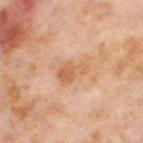Imaged during a routine full-body skin examination; the lesion was not biopsied and no histopathology is available.
Captured under cross-polarized illumination.
A 15 mm close-up tile from a total-body photography series done for melanoma screening.
The lesion is located on the right thigh.
Measured at roughly 3.5 mm in maximum diameter.
An algorithmic analysis of the crop reported an outline eccentricity of about 0.75 (0 = round, 1 = elongated). The analysis additionally found roughly 9 lightness units darker than nearby skin and a normalized lesion–skin contrast near 6. It also reported internal color variation of about 4 on a 0–10 scale and peripheral color asymmetry of about 1.5.
A female patient in their mid-50s.